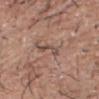workup: imaged on a skin check; not biopsied | size: ≈3 mm | body site: the head or neck | subject: male, roughly 65 years of age | acquisition: total-body-photography crop, ~15 mm field of view.